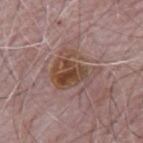Findings:
• follow-up — imaged on a skin check; not biopsied
• site — the mid back
• patient — male, roughly 65 years of age
• acquisition — ~15 mm tile from a whole-body skin photo
• TBP lesion metrics — a border-irregularity rating of about 6.5/10, internal color variation of about 8 on a 0–10 scale, and a peripheral color-asymmetry measure near 3; a nevus-likeness score of about 20/100
• lesion diameter — ~5.5 mm (longest diameter)